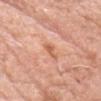Context: Located on the head or neck. Cropped from a total-body skin-imaging series; the visible field is about 15 mm. A male patient aged 73–77. The recorded lesion diameter is about 2.5 mm. Automated tile analysis of the lesion measured border irregularity of about 4 on a 0–10 scale, a within-lesion color-variation index near 0/10, and a peripheral color-asymmetry measure near 0. The software also gave a nevus-likeness score of about 0/100 and a detector confidence of about 100 out of 100 that the crop contains a lesion. Imaged with white-light lighting.